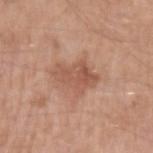This lesion was catalogued during total-body skin photography and was not selected for biopsy.
The lesion is on the arm.
Cropped from a total-body skin-imaging series; the visible field is about 15 mm.
A male subject, approximately 55 years of age.